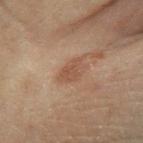{"biopsy_status": "not biopsied; imaged during a skin examination", "image": {"source": "total-body photography crop", "field_of_view_mm": 15}, "patient": {"sex": "male", "age_approx": 70}, "site": "leg"}